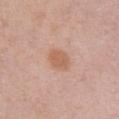Assessment: Captured during whole-body skin photography for melanoma surveillance; the lesion was not biopsied. Clinical summary: From the chest. This is a white-light tile. Cropped from a total-body skin-imaging series; the visible field is about 15 mm. A female subject aged 63 to 67. About 3 mm across.